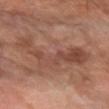Impression:
The lesion was tiled from a total-body skin photograph and was not biopsied.
Image and clinical context:
The lesion is located on the left forearm. Cropped from a total-body skin-imaging series; the visible field is about 15 mm. The subject is a male approximately 60 years of age. The tile uses cross-polarized illumination.